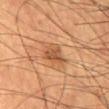Part of a total-body skin-imaging series; this lesion was reviewed on a skin check and was not flagged for biopsy.
A 15 mm close-up extracted from a 3D total-body photography capture.
The lesion-visualizer software estimated an eccentricity of roughly 0.2 and two-axis asymmetry of about 0.35. And it measured a lesion color around L≈47 a*≈21 b*≈33 in CIELAB and about 9 CIELAB-L* units darker than the surrounding skin. It also reported a border-irregularity rating of about 3/10, a color-variation rating of about 3/10, and a peripheral color-asymmetry measure near 1. The software also gave an automated nevus-likeness rating near 35 out of 100.
A male subject in their 60s.
The lesion's longest dimension is about 3 mm.